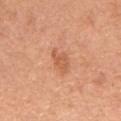follow-up — total-body-photography surveillance lesion; no biopsy
lesion size — ≈3 mm
image — ~15 mm crop, total-body skin-cancer survey
lighting — white-light
patient — male, aged 63 to 67
anatomic site — the front of the torso
TBP lesion metrics — a shape-asymmetry score of about 0.35 (0 = symmetric); a mean CIELAB color near L≈59 a*≈27 b*≈37, about 9 CIELAB-L* units darker than the surrounding skin, and a normalized border contrast of about 6; an automated nevus-likeness rating near 15 out of 100 and lesion-presence confidence of about 100/100